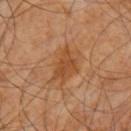Recorded during total-body skin imaging; not selected for excision or biopsy.
From the left upper arm.
Measured at roughly 4.5 mm in maximum diameter.
Cropped from a total-body skin-imaging series; the visible field is about 15 mm.
A male subject, about 60 years old.